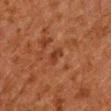Clinical impression:
This lesion was catalogued during total-body skin photography and was not selected for biopsy.
Clinical summary:
Automated tile analysis of the lesion measured a nevus-likeness score of about 0/100 and lesion-presence confidence of about 100/100. On the chest. A 15 mm close-up extracted from a 3D total-body photography capture. The patient is a male approximately 60 years of age.